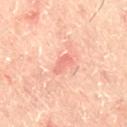workup: catalogued during a skin exam; not biopsied
lighting: cross-polarized
diameter: ≈3 mm
subject: male, about 50 years old
automated lesion analysis: an area of roughly 3 mm² and a shape-asymmetry score of about 0.35 (0 = symmetric); a border-irregularity index near 4/10 and a peripheral color-asymmetry measure near 0; a classifier nevus-likeness of about 0/100
site: the right thigh
acquisition: ~15 mm tile from a whole-body skin photo